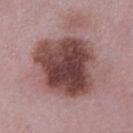Clinical impression: Part of a total-body skin-imaging series; this lesion was reviewed on a skin check and was not flagged for biopsy. Context: Longest diameter approximately 7.5 mm. Captured under white-light illumination. The subject is a male approximately 50 years of age. The lesion is located on the leg. A 15 mm crop from a total-body photograph taken for skin-cancer surveillance. Automated tile analysis of the lesion measured a footprint of about 40 mm², an outline eccentricity of about 0.4 (0 = round, 1 = elongated), and two-axis asymmetry of about 0.2. The analysis additionally found an average lesion color of about L≈44 a*≈21 b*≈20 (CIELAB), roughly 17 lightness units darker than nearby skin, and a lesion-to-skin contrast of about 12 (normalized; higher = more distinct).